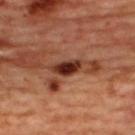No biopsy was performed on this lesion — it was imaged during a full skin examination and was not determined to be concerning.
The total-body-photography lesion software estimated a footprint of about 6 mm², a shape eccentricity near 0.9, and a shape-asymmetry score of about 0.25 (0 = symmetric). And it measured an average lesion color of about L≈33 a*≈25 b*≈28 (CIELAB), about 16 CIELAB-L* units darker than the surrounding skin, and a lesion-to-skin contrast of about 13 (normalized; higher = more distinct). The analysis additionally found a border-irregularity index near 3/10, a within-lesion color-variation index near 4/10, and radial color variation of about 1. It also reported an automated nevus-likeness rating near 10 out of 100 and a lesion-detection confidence of about 95/100.
The tile uses cross-polarized illumination.
The patient is a female approximately 45 years of age.
About 4 mm across.
A 15 mm crop from a total-body photograph taken for skin-cancer surveillance.
On the back.